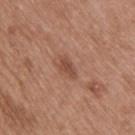Impression: Captured during whole-body skin photography for melanoma surveillance; the lesion was not biopsied.